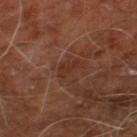Impression: Recorded during total-body skin imaging; not selected for excision or biopsy. Background: The recorded lesion diameter is about 3 mm. This is a cross-polarized tile. Cropped from a total-body skin-imaging series; the visible field is about 15 mm. The subject is a male aged around 60. The lesion is located on the right leg.